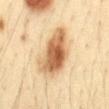Clinical impression:
The lesion was photographed on a routine skin check and not biopsied; there is no pathology result.
Clinical summary:
Captured under cross-polarized illumination. A male patient about 35 years old. Located on the abdomen. A roughly 15 mm field-of-view crop from a total-body skin photograph. The lesion-visualizer software estimated border irregularity of about 3 on a 0–10 scale and a peripheral color-asymmetry measure near 2.5. The software also gave a classifier nevus-likeness of about 100/100 and a lesion-detection confidence of about 100/100.